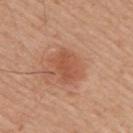The lesion was photographed on a routine skin check and not biopsied; there is no pathology result. A male patient, roughly 55 years of age. About 3.5 mm across. A 15 mm crop from a total-body photograph taken for skin-cancer surveillance. The lesion is located on the right upper arm. The tile uses white-light illumination. Automated image analysis of the tile measured an average lesion color of about L≈53 a*≈26 b*≈34 (CIELAB), a lesion–skin lightness drop of about 8, and a normalized lesion–skin contrast near 5.5. It also reported lesion-presence confidence of about 100/100.